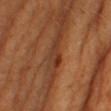Part of a total-body skin-imaging series; this lesion was reviewed on a skin check and was not flagged for biopsy. A female subject, aged 53 to 57. From the right lower leg. A roughly 15 mm field-of-view crop from a total-body skin photograph. The lesion's longest dimension is about 3 mm.